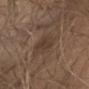The recorded lesion diameter is about 5 mm. Automated tile analysis of the lesion measured an automated nevus-likeness rating near 10 out of 100 and a lesion-detection confidence of about 80/100. The subject is a male in their 30s. The tile uses white-light illumination. A 15 mm close-up extracted from a 3D total-body photography capture. On the chest.